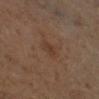Assessment:
Captured during whole-body skin photography for melanoma surveillance; the lesion was not biopsied.
Context:
The recorded lesion diameter is about 2.5 mm. The tile uses cross-polarized illumination. Automated image analysis of the tile measured internal color variation of about 1.5 on a 0–10 scale. The analysis additionally found an automated nevus-likeness rating near 0 out of 100 and a lesion-detection confidence of about 100/100. On the right lower leg. Cropped from a total-body skin-imaging series; the visible field is about 15 mm. The subject is a female roughly 50 years of age.